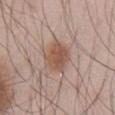notes: total-body-photography surveillance lesion; no biopsy
imaging modality: ~15 mm tile from a whole-body skin photo
site: the abdomen
subject: male, aged 53 to 57
lesion diameter: ~4 mm (longest diameter)